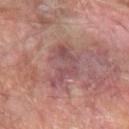Context:
A lesion tile, about 15 mm wide, cut from a 3D total-body photograph. Approximately 6 mm at its widest. The subject is a male aged 68 to 72. On the left forearm.